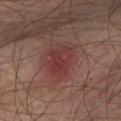{"biopsy_status": "not biopsied; imaged during a skin examination", "lesion_size": {"long_diameter_mm_approx": 4.0}, "image": {"source": "total-body photography crop", "field_of_view_mm": 15}, "site": "left lower leg", "lighting": "cross-polarized", "patient": {"sex": "male", "age_approx": 75}}